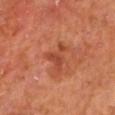Q: Was a biopsy performed?
A: catalogued during a skin exam; not biopsied